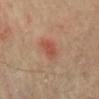Findings:
- biopsy status — no biopsy performed (imaged during a skin exam)
- size — ≈3 mm
- patient — male, about 65 years old
- image source — 15 mm crop, total-body photography
- image-analysis metrics — an area of roughly 5 mm², a shape eccentricity near 0.75, and a shape-asymmetry score of about 0.2 (0 = symmetric); a border-irregularity index near 2.5/10, a color-variation rating of about 2.5/10, and radial color variation of about 1
- location — the abdomen